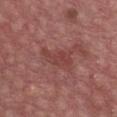Imaged during a routine full-body skin examination; the lesion was not biopsied and no histopathology is available. The total-body-photography lesion software estimated a lesion area of about 5.5 mm², a shape eccentricity near 0.9, and a symmetry-axis asymmetry near 0.5. The analysis additionally found a color-variation rating of about 1.5/10. It also reported a nevus-likeness score of about 0/100. Imaged with white-light lighting. A 15 mm close-up tile from a total-body photography series done for melanoma screening. Located on the chest. A male subject in their 60s.